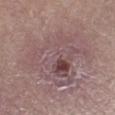Assessment:
The lesion was photographed on a routine skin check and not biopsied; there is no pathology result.
Acquisition and patient details:
A roughly 15 mm field-of-view crop from a total-body skin photograph. The lesion is located on the left lower leg. Automated image analysis of the tile measured a footprint of about 44 mm², an eccentricity of roughly 0.65, and a shape-asymmetry score of about 0.3 (0 = symmetric). The software also gave a nevus-likeness score of about 0/100 and lesion-presence confidence of about 100/100. Measured at roughly 9.5 mm in maximum diameter. A male patient aged 53–57.